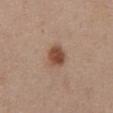Findings:
• size: ≈3 mm
• lighting: white-light illumination
• image source: ~15 mm crop, total-body skin-cancer survey
• location: the abdomen
• subject: male, approximately 60 years of age